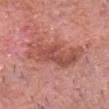Q: Was a biopsy performed?
A: imaged on a skin check; not biopsied
Q: Patient demographics?
A: male, in their 80s
Q: What is the imaging modality?
A: ~15 mm crop, total-body skin-cancer survey
Q: How large is the lesion?
A: ≈7.5 mm
Q: Illumination type?
A: white-light
Q: Where on the body is the lesion?
A: the head or neck